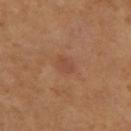Notes:
* workup: catalogued during a skin exam; not biopsied